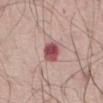This lesion was catalogued during total-body skin photography and was not selected for biopsy.
An algorithmic analysis of the crop reported a lesion area of about 6.5 mm² and a symmetry-axis asymmetry near 0.2. The analysis additionally found an automated nevus-likeness rating near 10 out of 100.
A male patient, roughly 60 years of age.
Imaged with white-light lighting.
A lesion tile, about 15 mm wide, cut from a 3D total-body photograph.
The recorded lesion diameter is about 3.5 mm.
The lesion is on the abdomen.